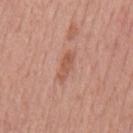Background:
A male patient, in their mid-50s. The total-body-photography lesion software estimated a symmetry-axis asymmetry near 0.2. The software also gave a peripheral color-asymmetry measure near 0.5. The lesion's longest dimension is about 3.5 mm. Located on the mid back. The tile uses white-light illumination. A lesion tile, about 15 mm wide, cut from a 3D total-body photograph.